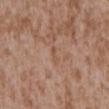Imaged during a routine full-body skin examination; the lesion was not biopsied and no histopathology is available.
This is a white-light tile.
The lesion is on the mid back.
Cropped from a whole-body photographic skin survey; the tile spans about 15 mm.
A male patient approximately 45 years of age.
The lesion's longest dimension is about 2.5 mm.